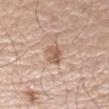Assessment: Part of a total-body skin-imaging series; this lesion was reviewed on a skin check and was not flagged for biopsy. Clinical summary: Cropped from a total-body skin-imaging series; the visible field is about 15 mm. On the right forearm. Measured at roughly 2.5 mm in maximum diameter. A male patient, aged around 60. Captured under white-light illumination.